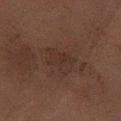Q: Is there a histopathology result?
A: catalogued during a skin exam; not biopsied
Q: How was this image acquired?
A: ~15 mm crop, total-body skin-cancer survey
Q: How was the tile lit?
A: cross-polarized
Q: What is the lesion's diameter?
A: about 4.5 mm
Q: Who is the patient?
A: male, aged around 80
Q: Lesion location?
A: the left forearm